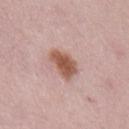* notes — catalogued during a skin exam; not biopsied
* site — the chest
* subject — female, about 40 years old
* TBP lesion metrics — an area of roughly 8 mm², an outline eccentricity of about 0.7 (0 = round, 1 = elongated), and a symmetry-axis asymmetry near 0.25; a lesion color around L≈55 a*≈22 b*≈28 in CIELAB, roughly 14 lightness units darker than nearby skin, and a normalized lesion–skin contrast near 10; a nevus-likeness score of about 95/100 and lesion-presence confidence of about 100/100
* diameter — about 3.5 mm
* imaging modality — total-body-photography crop, ~15 mm field of view